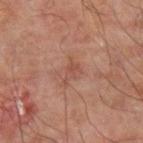Notes:
* biopsy status · imaged on a skin check; not biopsied
* illumination · cross-polarized
* imaging modality · total-body-photography crop, ~15 mm field of view
* location · the arm
* patient · male, aged 58–62